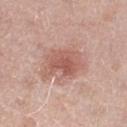workup = no biopsy performed (imaged during a skin exam)
lesion size = ≈3.5 mm
automated lesion analysis = a shape eccentricity near 0.7 and a symmetry-axis asymmetry near 0.35; a mean CIELAB color near L≈55 a*≈25 b*≈27, roughly 10 lightness units darker than nearby skin, and a normalized lesion–skin contrast near 6.5; a nevus-likeness score of about 5/100
acquisition = 15 mm crop, total-body photography
site = the right thigh
patient = female, approximately 60 years of age